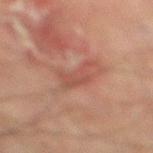{
  "biopsy_status": "not biopsied; imaged during a skin examination",
  "site": "right lower leg",
  "patient": {
    "sex": "male",
    "age_approx": 75
  },
  "image": {
    "source": "total-body photography crop",
    "field_of_view_mm": 15
  }
}